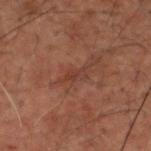No biopsy was performed on this lesion — it was imaged during a full skin examination and was not determined to be concerning. A close-up tile cropped from a whole-body skin photograph, about 15 mm across. Measured at roughly 3.5 mm in maximum diameter. The lesion is located on the head or neck. The subject is a male aged 58 to 62. This is a cross-polarized tile.